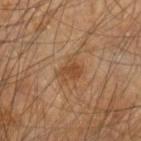Case summary:
- notes — total-body-photography surveillance lesion; no biopsy